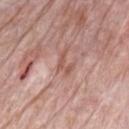Q: Is there a histopathology result?
A: imaged on a skin check; not biopsied
Q: What is the imaging modality?
A: ~15 mm tile from a whole-body skin photo
Q: Patient demographics?
A: male, aged approximately 70
Q: What is the anatomic site?
A: the chest
Q: Lesion size?
A: ~3 mm (longest diameter)
Q: Illumination type?
A: white-light illumination